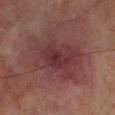Clinical impression: No biopsy was performed on this lesion — it was imaged during a full skin examination and was not determined to be concerning. Acquisition and patient details: About 6 mm across. On the left lower leg. A close-up tile cropped from a whole-body skin photograph, about 15 mm across. Imaged with cross-polarized lighting. The patient is a male approximately 70 years of age.